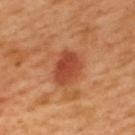workup: catalogued during a skin exam; not biopsied | image source: total-body-photography crop, ~15 mm field of view | anatomic site: the upper back | subject: male, aged around 50 | lesion size: ≈4 mm | illumination: cross-polarized.